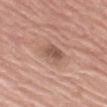Case summary:
* biopsy status — total-body-photography surveillance lesion; no biopsy
* acquisition — 15 mm crop, total-body photography
* subject — male, approximately 80 years of age
* anatomic site — the left thigh
* illumination — white-light illumination
* image-analysis metrics — a footprint of about 4 mm², an outline eccentricity of about 0.75 (0 = round, 1 = elongated), and a symmetry-axis asymmetry near 0.2; a border-irregularity index near 1.5/10 and radial color variation of about 1; an automated nevus-likeness rating near 0 out of 100
* lesion diameter — ≈2.5 mm